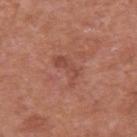Q: Is there a histopathology result?
A: catalogued during a skin exam; not biopsied
Q: Where on the body is the lesion?
A: the left upper arm
Q: Who is the patient?
A: male, aged around 65
Q: What is the lesion's diameter?
A: ~4 mm (longest diameter)
Q: Illumination type?
A: white-light
Q: What kind of image is this?
A: ~15 mm tile from a whole-body skin photo